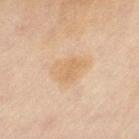Q: Was this lesion biopsied?
A: no biopsy performed (imaged during a skin exam)
Q: What is the imaging modality?
A: ~15 mm tile from a whole-body skin photo
Q: Automated lesion metrics?
A: a shape eccentricity near 0.7; a lesion–skin lightness drop of about 6 and a lesion-to-skin contrast of about 5.5 (normalized; higher = more distinct); a border-irregularity index near 3/10, a color-variation rating of about 2/10, and a peripheral color-asymmetry measure near 0.5; an automated nevus-likeness rating near 10 out of 100 and a lesion-detection confidence of about 100/100
Q: What is the anatomic site?
A: the leg
Q: How was the tile lit?
A: cross-polarized
Q: Who is the patient?
A: female, aged 58 to 62
Q: How large is the lesion?
A: about 4 mm